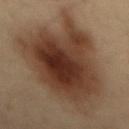workup: no biopsy performed (imaged during a skin exam) | subject: male, aged around 35 | location: the mid back | lesion diameter: about 15 mm | image: ~15 mm tile from a whole-body skin photo.